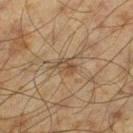This lesion was catalogued during total-body skin photography and was not selected for biopsy.
Captured under cross-polarized illumination.
The total-body-photography lesion software estimated a nevus-likeness score of about 0/100 and a detector confidence of about 85 out of 100 that the crop contains a lesion.
From the left thigh.
Approximately 2.5 mm at its widest.
A roughly 15 mm field-of-view crop from a total-body skin photograph.
A male patient roughly 60 years of age.